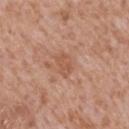biopsy status: catalogued during a skin exam; not biopsied
anatomic site: the back
patient: male, in their mid-60s
automated metrics: a lesion area of about 4.5 mm²; an average lesion color of about L≈55 a*≈23 b*≈32 (CIELAB) and roughly 7 lightness units darker than nearby skin; a border-irregularity rating of about 2.5/10, a color-variation rating of about 1.5/10, and radial color variation of about 0.5; an automated nevus-likeness rating near 0 out of 100 and lesion-presence confidence of about 100/100
imaging modality: ~15 mm crop, total-body skin-cancer survey